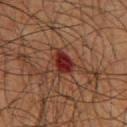From the chest. A 15 mm close-up tile from a total-body photography series done for melanoma screening. This is a cross-polarized tile. A male subject aged around 65. Measured at roughly 3.5 mm in maximum diameter.